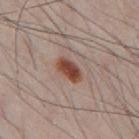No biopsy was performed on this lesion — it was imaged during a full skin examination and was not determined to be concerning. A male patient, approximately 35 years of age. The recorded lesion diameter is about 3.5 mm. Automated image analysis of the tile measured a border-irregularity rating of about 2/10. The software also gave a nevus-likeness score of about 100/100 and lesion-presence confidence of about 100/100. A region of skin cropped from a whole-body photographic capture, roughly 15 mm wide. Located on the mid back.